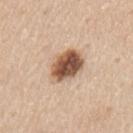Clinical impression: The lesion was tiled from a total-body skin photograph and was not biopsied. Context: A roughly 15 mm field-of-view crop from a total-body skin photograph. A male patient in their mid-70s. The lesion's longest dimension is about 4.5 mm. The total-body-photography lesion software estimated a footprint of about 12 mm². The tile uses white-light illumination. The lesion is located on the arm.